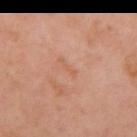Imaged during a routine full-body skin examination; the lesion was not biopsied and no histopathology is available.
Longest diameter approximately 2.5 mm.
A female patient aged around 55.
The lesion is located on the right upper arm.
An algorithmic analysis of the crop reported a classifier nevus-likeness of about 0/100 and lesion-presence confidence of about 100/100.
A lesion tile, about 15 mm wide, cut from a 3D total-body photograph.
Imaged with cross-polarized lighting.